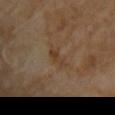This lesion was catalogued during total-body skin photography and was not selected for biopsy. A male subject aged 68–72. A 15 mm close-up extracted from a 3D total-body photography capture. Automated image analysis of the tile measured a lesion area of about 3 mm² and a symmetry-axis asymmetry near 0.4. The analysis additionally found a border-irregularity rating of about 4/10, internal color variation of about 2 on a 0–10 scale, and a peripheral color-asymmetry measure near 0.5. It also reported a nevus-likeness score of about 0/100. About 2.5 mm across. The tile uses cross-polarized illumination. On the right upper arm.